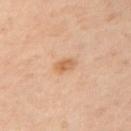| key | value |
|---|---|
| anatomic site | the left upper arm |
| patient | female, aged 38 to 42 |
| tile lighting | cross-polarized |
| automated metrics | a lesion area of about 3.5 mm² and an outline eccentricity of about 0.75 (0 = round, 1 = elongated); an average lesion color of about L≈63 a*≈22 b*≈37 (CIELAB), a lesion–skin lightness drop of about 9, and a normalized lesion–skin contrast near 7 |
| image | 15 mm crop, total-body photography |
| lesion diameter | ~2.5 mm (longest diameter) |